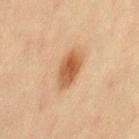Captured during whole-body skin photography for melanoma surveillance; the lesion was not biopsied. A close-up tile cropped from a whole-body skin photograph, about 15 mm across. A female subject, in their mid-40s. An algorithmic analysis of the crop reported a footprint of about 8.5 mm² and a shape-asymmetry score of about 0.15 (0 = symmetric). The software also gave a mean CIELAB color near L≈50 a*≈21 b*≈34, roughly 12 lightness units darker than nearby skin, and a lesion-to-skin contrast of about 9 (normalized; higher = more distinct). It also reported a border-irregularity rating of about 2/10 and a within-lesion color-variation index near 4.5/10. The analysis additionally found a classifier nevus-likeness of about 100/100. The lesion's longest dimension is about 5 mm. Located on the leg.